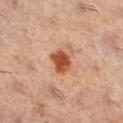Q: Was a biopsy performed?
A: imaged on a skin check; not biopsied
Q: How was the tile lit?
A: cross-polarized illumination
Q: What is the imaging modality?
A: ~15 mm tile from a whole-body skin photo
Q: Who is the patient?
A: female, in their 60s
Q: Lesion size?
A: ≈2.5 mm
Q: What did automated image analysis measure?
A: an area of roughly 5.5 mm² and a symmetry-axis asymmetry near 0.2; a lesion color around L≈53 a*≈29 b*≈38 in CIELAB, a lesion–skin lightness drop of about 16, and a normalized border contrast of about 11.5; a border-irregularity index near 2/10 and radial color variation of about 1
Q: What is the anatomic site?
A: the right lower leg